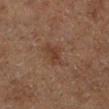Findings:
* acquisition · total-body-photography crop, ~15 mm field of view
* automated metrics · an area of roughly 5.5 mm², an outline eccentricity of about 0.8 (0 = round, 1 = elongated), and two-axis asymmetry of about 0.3; a lesion color around L≈30 a*≈15 b*≈22 in CIELAB and a normalized lesion–skin contrast near 5.5; border irregularity of about 3.5 on a 0–10 scale and a color-variation rating of about 2/10; a nevus-likeness score of about 0/100
* subject · male, in their mid- to late 70s
* location · the leg
* diameter · about 3.5 mm